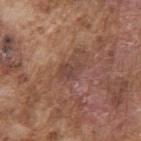Q: Was a biopsy performed?
A: imaged on a skin check; not biopsied
Q: What is the imaging modality?
A: 15 mm crop, total-body photography
Q: Who is the patient?
A: male, approximately 75 years of age
Q: What lighting was used for the tile?
A: white-light
Q: What is the lesion's diameter?
A: ~3 mm (longest diameter)
Q: Where on the body is the lesion?
A: the right upper arm
Q: Automated lesion metrics?
A: a border-irregularity rating of about 2.5/10, a within-lesion color-variation index near 2/10, and a peripheral color-asymmetry measure near 0.5